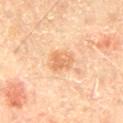Case summary:
- biopsy status · total-body-photography surveillance lesion; no biopsy
- TBP lesion metrics · a lesion color around L≈69 a*≈23 b*≈40 in CIELAB, a lesion–skin lightness drop of about 9, and a lesion-to-skin contrast of about 6 (normalized; higher = more distinct); border irregularity of about 2.5 on a 0–10 scale, internal color variation of about 3 on a 0–10 scale, and radial color variation of about 1
- tile lighting · cross-polarized illumination
- lesion diameter · ≈3.5 mm
- image · ~15 mm crop, total-body skin-cancer survey
- subject · male, in their mid-60s
- anatomic site · the lower back